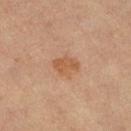Assessment: The lesion was photographed on a routine skin check and not biopsied; there is no pathology result. Clinical summary: A female patient, approximately 65 years of age. This is a cross-polarized tile. The lesion-visualizer software estimated a footprint of about 5.5 mm², an outline eccentricity of about 0.65 (0 = round, 1 = elongated), and a symmetry-axis asymmetry near 0.3. And it measured an average lesion color of about L≈53 a*≈21 b*≈34 (CIELAB), roughly 8 lightness units darker than nearby skin, and a normalized lesion–skin contrast near 6.5. The software also gave a classifier nevus-likeness of about 45/100. The recorded lesion diameter is about 3 mm. Cropped from a whole-body photographic skin survey; the tile spans about 15 mm. On the left leg.